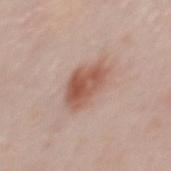biopsy_status: not biopsied; imaged during a skin examination
patient:
  sex: male
  age_approx: 50
site: mid back
lighting: white-light
automated_metrics:
  cielab_L: 55
  cielab_a: 22
  cielab_b: 28
  vs_skin_darker_L: 12.0
  vs_skin_contrast_norm: 8.5
  border_irregularity_0_10: 3.0
  peripheral_color_asymmetry: 2.0
  nevus_likeness_0_100: 95
  lesion_detection_confidence_0_100: 100
image:
  source: total-body photography crop
  field_of_view_mm: 15
lesion_size:
  long_diameter_mm_approx: 5.0